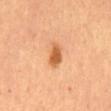The lesion was tiled from a total-body skin photograph and was not biopsied. Imaged with cross-polarized lighting. A 15 mm crop from a total-body photograph taken for skin-cancer surveillance. The subject is female. Longest diameter approximately 3 mm. Located on the abdomen. An algorithmic analysis of the crop reported a footprint of about 5.5 mm², an eccentricity of roughly 0.8, and a symmetry-axis asymmetry near 0.3. The analysis additionally found an average lesion color of about L≈62 a*≈27 b*≈43 (CIELAB) and a lesion-to-skin contrast of about 8.5 (normalized; higher = more distinct). It also reported a border-irregularity index near 2.5/10, internal color variation of about 3 on a 0–10 scale, and a peripheral color-asymmetry measure near 1. And it measured a classifier nevus-likeness of about 100/100 and lesion-presence confidence of about 100/100.